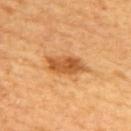<lesion>
<biopsy_status>not biopsied; imaged during a skin examination</biopsy_status>
<site>upper back</site>
<patient>
  <sex>female</sex>
  <age_approx>35</age_approx>
</patient>
<lighting>cross-polarized</lighting>
<image>
  <source>total-body photography crop</source>
  <field_of_view_mm>15</field_of_view_mm>
</image>
<lesion_size>
  <long_diameter_mm_approx>5.0</long_diameter_mm_approx>
</lesion_size>
</lesion>